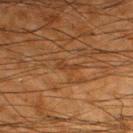Part of a total-body skin-imaging series; this lesion was reviewed on a skin check and was not flagged for biopsy.
The lesion's longest dimension is about 2.5 mm.
A 15 mm close-up tile from a total-body photography series done for melanoma screening.
A male subject, aged 63–67.
The tile uses cross-polarized illumination.
Located on the left lower leg.